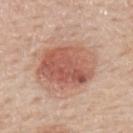{"biopsy_status": "not biopsied; imaged during a skin examination", "image": {"source": "total-body photography crop", "field_of_view_mm": 15}, "site": "upper back", "patient": {"sex": "male", "age_approx": 60}, "lighting": "white-light"}